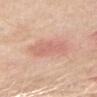This is a white-light tile.
Located on the right upper arm.
A female patient roughly 70 years of age.
A lesion tile, about 15 mm wide, cut from a 3D total-body photograph.
About 4 mm across.